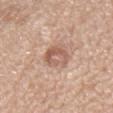Clinical impression: The lesion was photographed on a routine skin check and not biopsied; there is no pathology result. Acquisition and patient details: Imaged with white-light lighting. About 4 mm across. A male patient about 75 years old. Located on the chest. A 15 mm close-up extracted from a 3D total-body photography capture.